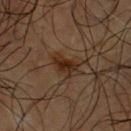Impression: The lesion was tiled from a total-body skin photograph and was not biopsied. Image and clinical context: The tile uses cross-polarized illumination. An algorithmic analysis of the crop reported a lesion color around L≈23 a*≈17 b*≈25 in CIELAB, a lesion–skin lightness drop of about 10, and a normalized border contrast of about 11.5. The analysis additionally found a border-irregularity rating of about 4/10 and radial color variation of about 0. And it measured a nevus-likeness score of about 70/100 and a detector confidence of about 100 out of 100 that the crop contains a lesion. Located on the front of the torso. A male patient, roughly 60 years of age. Cropped from a whole-body photographic skin survey; the tile spans about 15 mm.